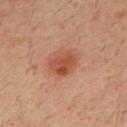Assessment:
Part of a total-body skin-imaging series; this lesion was reviewed on a skin check and was not flagged for biopsy.
Acquisition and patient details:
A male subject, aged approximately 30. Measured at roughly 4 mm in maximum diameter. The lesion is on the mid back. A roughly 15 mm field-of-view crop from a total-body skin photograph. The total-body-photography lesion software estimated a mean CIELAB color near L≈49 a*≈27 b*≈32, a lesion–skin lightness drop of about 10, and a normalized border contrast of about 7.5. The analysis additionally found a border-irregularity index near 2/10, a within-lesion color-variation index near 4.5/10, and a peripheral color-asymmetry measure near 1.5. It also reported a nevus-likeness score of about 100/100 and a lesion-detection confidence of about 100/100.